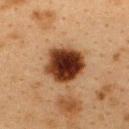workup = catalogued during a skin exam; not biopsied | tile lighting = cross-polarized illumination | patient = female, aged 38–42 | body site = the upper back | image source = 15 mm crop, total-body photography.